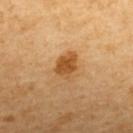Recorded during total-body skin imaging; not selected for excision or biopsy.
Cropped from a whole-body photographic skin survey; the tile spans about 15 mm.
From the upper back.
A female patient, roughly 55 years of age.
The recorded lesion diameter is about 3.5 mm.